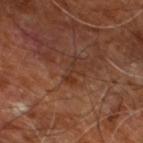Acquisition and patient details: This is a cross-polarized tile. The lesion is located on the right leg. Automated tile analysis of the lesion measured an outline eccentricity of about 0.95 (0 = round, 1 = elongated) and a symmetry-axis asymmetry near 0.55. It also reported a border-irregularity index near 7/10 and a color-variation rating of about 0/10. And it measured a classifier nevus-likeness of about 0/100 and lesion-presence confidence of about 100/100. A male patient aged 58 to 62. A 15 mm crop from a total-body photograph taken for skin-cancer surveillance. About 3.5 mm across.